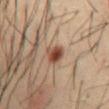anatomic site = the abdomen; lesion diameter = ~2.5 mm (longest diameter); acquisition = ~15 mm tile from a whole-body skin photo; tile lighting = cross-polarized; subject = male, aged 38 to 42.